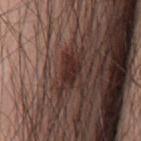Q: Is there a histopathology result?
A: catalogued during a skin exam; not biopsied
Q: Lesion size?
A: ≈5 mm
Q: Lesion location?
A: the back
Q: How was this image acquired?
A: ~15 mm crop, total-body skin-cancer survey
Q: What are the patient's age and sex?
A: male, aged approximately 60
Q: Automated lesion metrics?
A: a lesion area of about 8 mm², an outline eccentricity of about 0.9 (0 = round, 1 = elongated), and a symmetry-axis asymmetry near 0.35; a border-irregularity rating of about 5/10, a within-lesion color-variation index near 3/10, and a peripheral color-asymmetry measure near 1
Q: How was the tile lit?
A: white-light